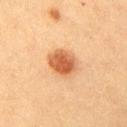The lesion was tiled from a total-body skin photograph and was not biopsied. The recorded lesion diameter is about 3.5 mm. Located on the chest. A 15 mm crop from a total-body photograph taken for skin-cancer surveillance. A female patient in their 40s.